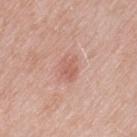<record>
  <site>left thigh</site>
  <lesion_size>
    <long_diameter_mm_approx>3.0</long_diameter_mm_approx>
  </lesion_size>
  <image>
    <source>total-body photography crop</source>
    <field_of_view_mm>15</field_of_view_mm>
  </image>
  <patient>
    <sex>female</sex>
    <age_approx>60</age_approx>
  </patient>
  <lighting>white-light</lighting>
  <automated_metrics>
    <cielab_L>60</cielab_L>
    <cielab_a>24</cielab_a>
    <cielab_b>28</cielab_b>
    <vs_skin_darker_L>8.0</vs_skin_darker_L>
    <vs_skin_contrast_norm>5.5</vs_skin_contrast_norm>
    <nevus_likeness_0_100>10</nevus_likeness_0_100>
    <lesion_detection_confidence_0_100>100</lesion_detection_confidence_0_100>
  </automated_metrics>
</record>